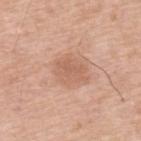| key | value |
|---|---|
| workup | no biopsy performed (imaged during a skin exam) |
| anatomic site | the back |
| subject | male, aged around 70 |
| image | 15 mm crop, total-body photography |